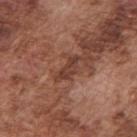Findings:
* notes: catalogued during a skin exam; not biopsied
* patient: male, aged around 75
* tile lighting: white-light illumination
* body site: the right upper arm
* diameter: ≈3.5 mm
* imaging modality: 15 mm crop, total-body photography